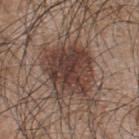The lesion was tiled from a total-body skin photograph and was not biopsied.
The lesion is on the upper back.
Automated tile analysis of the lesion measured an area of roughly 21 mm². And it measured about 12 CIELAB-L* units darker than the surrounding skin and a lesion-to-skin contrast of about 10 (normalized; higher = more distinct). The analysis additionally found a within-lesion color-variation index near 5/10 and a peripheral color-asymmetry measure near 1.5. The analysis additionally found a classifier nevus-likeness of about 40/100 and a lesion-detection confidence of about 100/100.
Imaged with white-light lighting.
This image is a 15 mm lesion crop taken from a total-body photograph.
A male subject, aged approximately 45.